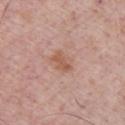The tile uses white-light illumination. This image is a 15 mm lesion crop taken from a total-body photograph. A male subject, in their mid-50s.